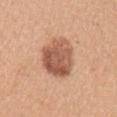biopsy_status: not biopsied; imaged during a skin examination
site: left upper arm
patient:
  sex: female
  age_approx: 30
lesion_size:
  long_diameter_mm_approx: 5.5
lighting: white-light
image:
  source: total-body photography crop
  field_of_view_mm: 15